The lesion-visualizer software estimated internal color variation of about 0 on a 0–10 scale. The tile uses white-light illumination. The lesion is on the arm. The lesion's longest dimension is about 1.5 mm. Cropped from a total-body skin-imaging series; the visible field is about 15 mm. A male subject, aged 53–57.
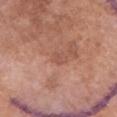Findings:
• pathology · a solar lentigo (benign)